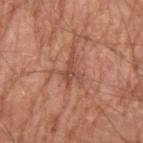Impression:
The lesion was photographed on a routine skin check and not biopsied; there is no pathology result.
Context:
Automated tile analysis of the lesion measured an area of roughly 4 mm², an outline eccentricity of about 0.8 (0 = round, 1 = elongated), and two-axis asymmetry of about 0.7. It also reported a border-irregularity index near 8.5/10, a color-variation rating of about 1/10, and radial color variation of about 0. A close-up tile cropped from a whole-body skin photograph, about 15 mm across. A male patient, aged 63 to 67. From the left upper arm. The tile uses white-light illumination. Measured at roughly 3.5 mm in maximum diameter.